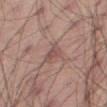Part of a total-body skin-imaging series; this lesion was reviewed on a skin check and was not flagged for biopsy. A male patient, aged 43 to 47. Cropped from a whole-body photographic skin survey; the tile spans about 15 mm. Approximately 3 mm at its widest. The lesion-visualizer software estimated a lesion area of about 4.5 mm² and an outline eccentricity of about 0.8 (0 = round, 1 = elongated). It also reported a within-lesion color-variation index near 1.5/10 and peripheral color asymmetry of about 0.5. It also reported a classifier nevus-likeness of about 0/100. The lesion is located on the right thigh. The tile uses white-light illumination.